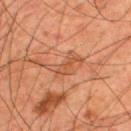Clinical impression:
Recorded during total-body skin imaging; not selected for excision or biopsy.
Background:
A male subject, in their mid-40s. Cropped from a whole-body photographic skin survey; the tile spans about 15 mm. The lesion is located on the back.